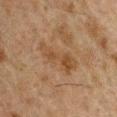Impression:
Captured during whole-body skin photography for melanoma surveillance; the lesion was not biopsied.
Context:
A male patient aged approximately 50. Cropped from a total-body skin-imaging series; the visible field is about 15 mm. The lesion is located on the left upper arm. Captured under cross-polarized illumination.